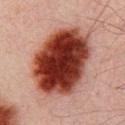Assessment:
The lesion was photographed on a routine skin check and not biopsied; there is no pathology result.
Context:
A male subject about 30 years old. An algorithmic analysis of the crop reported an average lesion color of about L≈31 a*≈26 b*≈25 (CIELAB), a lesion–skin lightness drop of about 22, and a normalized lesion–skin contrast near 18. It also reported a border-irregularity rating of about 1.5/10 and a within-lesion color-variation index near 8/10. Captured under cross-polarized illumination. Located on the chest. A region of skin cropped from a whole-body photographic capture, roughly 15 mm wide. The recorded lesion diameter is about 8.5 mm.